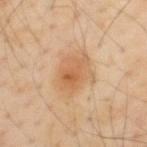patient = male, aged 53 to 57
acquisition = ~15 mm tile from a whole-body skin photo
anatomic site = the upper back